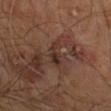anatomic site: the leg | automated metrics: a lesion area of about 3.5 mm², an outline eccentricity of about 0.9 (0 = round, 1 = elongated), and a shape-asymmetry score of about 0.45 (0 = symmetric) | image: 15 mm crop, total-body photography | illumination: cross-polarized | patient: male, aged approximately 60 | diameter: ~3 mm (longest diameter).